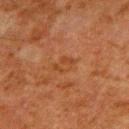illumination = cross-polarized | acquisition = ~15 mm crop, total-body skin-cancer survey | diameter = ≈3 mm | body site = the right upper arm | image-analysis metrics = a border-irregularity rating of about 4/10, internal color variation of about 3 on a 0–10 scale, and a peripheral color-asymmetry measure near 1 | subject = male, aged approximately 80.